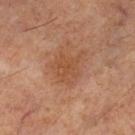Assessment: Part of a total-body skin-imaging series; this lesion was reviewed on a skin check and was not flagged for biopsy. Clinical summary: On the leg. A male patient aged around 60. A region of skin cropped from a whole-body photographic capture, roughly 15 mm wide.